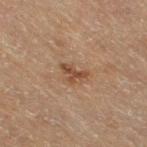No biopsy was performed on this lesion — it was imaged during a full skin examination and was not determined to be concerning. A 15 mm crop from a total-body photograph taken for skin-cancer surveillance. Captured under cross-polarized illumination. About 3 mm across. From the right thigh. The patient is a female roughly 55 years of age.